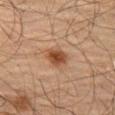No biopsy was performed on this lesion — it was imaged during a full skin examination and was not determined to be concerning.